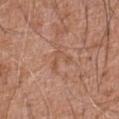| field | value |
|---|---|
| notes | catalogued during a skin exam; not biopsied |
| automated metrics | a footprint of about 3.5 mm², an outline eccentricity of about 0.75 (0 = round, 1 = elongated), and a symmetry-axis asymmetry near 0.7; an average lesion color of about L≈53 a*≈22 b*≈31 (CIELAB) and roughly 6 lightness units darker than nearby skin; a nevus-likeness score of about 0/100 and lesion-presence confidence of about 95/100 |
| lesion size | ≈3 mm |
| acquisition | total-body-photography crop, ~15 mm field of view |
| tile lighting | white-light illumination |
| body site | the abdomen |
| patient | male, in their mid-70s |